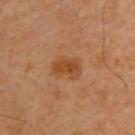{
  "patient": {
    "sex": "male",
    "age_approx": 45
  },
  "site": "upper back",
  "image": {
    "source": "total-body photography crop",
    "field_of_view_mm": 15
  },
  "lighting": "cross-polarized"
}